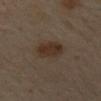Cropped from a whole-body photographic skin survey; the tile spans about 15 mm.
The tile uses cross-polarized illumination.
The lesion is located on the mid back.
A male patient in their mid-60s.
Longest diameter approximately 4 mm.